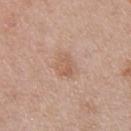site = the right upper arm | subject = female, about 50 years old | image source = total-body-photography crop, ~15 mm field of view | diameter = ~3 mm (longest diameter) | illumination = white-light.